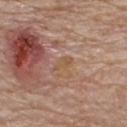Part of a total-body skin-imaging series; this lesion was reviewed on a skin check and was not flagged for biopsy.
A close-up tile cropped from a whole-body skin photograph, about 15 mm across.
On the upper back.
This is a white-light tile.
The lesion-visualizer software estimated a lesion color around L≈54 a*≈18 b*≈32 in CIELAB. And it measured a border-irregularity rating of about 3/10.
The recorded lesion diameter is about 2.5 mm.
The patient is a male in their 80s.